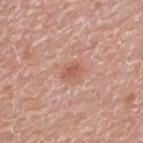Assessment:
The lesion was photographed on a routine skin check and not biopsied; there is no pathology result.
Clinical summary:
A male subject, roughly 75 years of age. This is a white-light tile. A close-up tile cropped from a whole-body skin photograph, about 15 mm across. Automated image analysis of the tile measured a footprint of about 3.5 mm², a shape eccentricity near 0.7, and two-axis asymmetry of about 0.2. The analysis additionally found border irregularity of about 2 on a 0–10 scale and internal color variation of about 1.5 on a 0–10 scale. It also reported a lesion-detection confidence of about 100/100. Longest diameter approximately 2.5 mm. On the upper back.